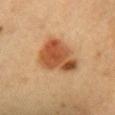Case summary:
– follow-up · total-body-photography surveillance lesion; no biopsy
– subject · female, aged 28 to 32
– anatomic site · the chest
– imaging modality · total-body-photography crop, ~15 mm field of view
– illumination · cross-polarized illumination
– diameter · ≈5.5 mm